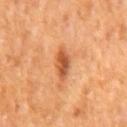Imaged during a routine full-body skin examination; the lesion was not biopsied and no histopathology is available.
On the mid back.
This is a cross-polarized tile.
Cropped from a whole-body photographic skin survey; the tile spans about 15 mm.
The subject is a male in their mid- to late 60s.
An algorithmic analysis of the crop reported a mean CIELAB color near L≈53 a*≈28 b*≈40, about 13 CIELAB-L* units darker than the surrounding skin, and a lesion-to-skin contrast of about 8.5 (normalized; higher = more distinct). And it measured border irregularity of about 2.5 on a 0–10 scale, a within-lesion color-variation index near 4.5/10, and peripheral color asymmetry of about 1.5. And it measured a nevus-likeness score of about 70/100.